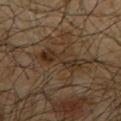workup = total-body-photography surveillance lesion; no biopsy
patient = male, approximately 65 years of age
acquisition = ~15 mm tile from a whole-body skin photo
size = about 5.5 mm
site = the upper back